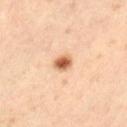biopsy status: imaged on a skin check; not biopsied
body site: the leg
diameter: ≈2 mm
acquisition: ~15 mm crop, total-body skin-cancer survey
lighting: cross-polarized
patient: male, in their mid-60s